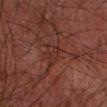biopsy status=total-body-photography surveillance lesion; no biopsy
subject=male, about 65 years old
lesion diameter=≈3 mm
image=15 mm crop, total-body photography
location=the left forearm